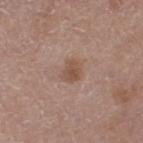follow-up: no biopsy performed (imaged during a skin exam)
size: about 2.5 mm
illumination: white-light illumination
subject: male, roughly 75 years of age
site: the left thigh
imaging modality: ~15 mm crop, total-body skin-cancer survey